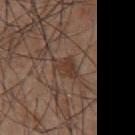follow-up: imaged on a skin check; not biopsied | lighting: white-light illumination | subject: male, roughly 55 years of age | acquisition: ~15 mm crop, total-body skin-cancer survey | lesion diameter: ≈3 mm | image-analysis metrics: a lesion–skin lightness drop of about 7 and a lesion-to-skin contrast of about 7 (normalized; higher = more distinct); a border-irregularity index near 4/10, a color-variation rating of about 1.5/10, and a peripheral color-asymmetry measure near 0.5; an automated nevus-likeness rating near 0 out of 100 and lesion-presence confidence of about 95/100 | site: the abdomen.